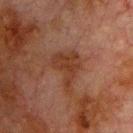The lesion was photographed on a routine skin check and not biopsied; there is no pathology result. About 4.5 mm across. Imaged with cross-polarized lighting. A male patient, in their 80s. From the chest. A 15 mm crop from a total-body photograph taken for skin-cancer surveillance.